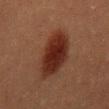biopsy status: imaged on a skin check; not biopsied
subject: male, about 40 years old
image source: 15 mm crop, total-body photography
site: the mid back